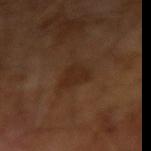No biopsy was performed on this lesion — it was imaged during a full skin examination and was not determined to be concerning. The subject is a male in their mid- to late 60s. A roughly 15 mm field-of-view crop from a total-body skin photograph.